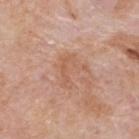The lesion was photographed on a routine skin check and not biopsied; there is no pathology result.
Captured under white-light illumination.
This image is a 15 mm lesion crop taken from a total-body photograph.
The patient is a male aged 73–77.
The lesion is on the back.